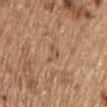Notes:
– notes: imaged on a skin check; not biopsied
– patient: male, aged approximately 70
– image: 15 mm crop, total-body photography
– body site: the upper back
– illumination: white-light illumination
– lesion diameter: about 3 mm
– image-analysis metrics: a lesion color around L≈53 a*≈19 b*≈33 in CIELAB, about 6 CIELAB-L* units darker than the surrounding skin, and a normalized lesion–skin contrast near 4.5; internal color variation of about 2.5 on a 0–10 scale and a peripheral color-asymmetry measure near 1